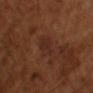A male subject, aged around 65. A close-up tile cropped from a whole-body skin photograph, about 15 mm across.